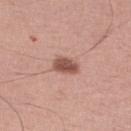notes — imaged on a skin check; not biopsied | site — the right lower leg | acquisition — total-body-photography crop, ~15 mm field of view | lighting — white-light illumination | automated metrics — a lesion color around L≈53 a*≈23 b*≈25 in CIELAB, about 14 CIELAB-L* units darker than the surrounding skin, and a normalized lesion–skin contrast near 9 | patient — male, aged 48 to 52.